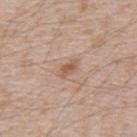Clinical impression:
Imaged during a routine full-body skin examination; the lesion was not biopsied and no histopathology is available.
Acquisition and patient details:
A 15 mm crop from a total-body photograph taken for skin-cancer surveillance. A male subject about 50 years old. Imaged with white-light lighting. Longest diameter approximately 2.5 mm. The lesion is located on the mid back.